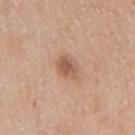Imaged during a routine full-body skin examination; the lesion was not biopsied and no histopathology is available. A male patient aged 78–82. A roughly 15 mm field-of-view crop from a total-body skin photograph. Automated tile analysis of the lesion measured an area of roughly 5.5 mm² and an outline eccentricity of about 0.45 (0 = round, 1 = elongated). And it measured an average lesion color of about L≈57 a*≈21 b*≈31 (CIELAB), a lesion–skin lightness drop of about 10, and a normalized border contrast of about 7. Located on the right upper arm.